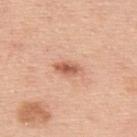Notes:
• follow-up: no biopsy performed (imaged during a skin exam)
• lesion diameter: ~3 mm (longest diameter)
• anatomic site: the upper back
• patient: male, approximately 50 years of age
• image: ~15 mm crop, total-body skin-cancer survey